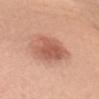| field | value |
|---|---|
| workup | total-body-photography surveillance lesion; no biopsy |
| tile lighting | white-light illumination |
| site | the left upper arm |
| subject | male, aged approximately 50 |
| lesion diameter | ≈5.5 mm |
| acquisition | ~15 mm tile from a whole-body skin photo |